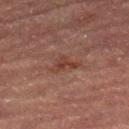Q: Is there a histopathology result?
A: total-body-photography surveillance lesion; no biopsy
Q: What is the lesion's diameter?
A: about 3 mm
Q: What is the anatomic site?
A: the right lower leg
Q: What kind of image is this?
A: ~15 mm tile from a whole-body skin photo
Q: What did automated image analysis measure?
A: an average lesion color of about L≈33 a*≈19 b*≈23 (CIELAB), about 6 CIELAB-L* units darker than the surrounding skin, and a normalized border contrast of about 6.5; a color-variation rating of about 2/10; a classifier nevus-likeness of about 5/100 and a lesion-detection confidence of about 100/100
Q: What are the patient's age and sex?
A: female, roughly 70 years of age